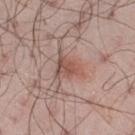acquisition = total-body-photography crop, ~15 mm field of view
anatomic site = the right thigh
automated lesion analysis = a lesion color around L≈52 a*≈20 b*≈25 in CIELAB and a normalized border contrast of about 7; border irregularity of about 5.5 on a 0–10 scale and a within-lesion color-variation index near 3/10; an automated nevus-likeness rating near 85 out of 100 and a detector confidence of about 100 out of 100 that the crop contains a lesion
patient = male, about 50 years old
lighting = white-light